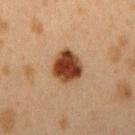No biopsy was performed on this lesion — it was imaged during a full skin examination and was not determined to be concerning.
The lesion is on the right upper arm.
A female subject, roughly 40 years of age.
This image is a 15 mm lesion crop taken from a total-body photograph.
Imaged with cross-polarized lighting.
The lesion's longest dimension is about 3.5 mm.